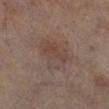Assessment:
The lesion was photographed on a routine skin check and not biopsied; there is no pathology result.
Image and clinical context:
From the left lower leg. A 15 mm close-up extracted from a 3D total-body photography capture. The recorded lesion diameter is about 4.5 mm. This is a cross-polarized tile. Automated image analysis of the tile measured a lesion area of about 14 mm², an eccentricity of roughly 0.6, and two-axis asymmetry of about 0.25. The software also gave an average lesion color of about L≈36 a*≈13 b*≈19 (CIELAB) and roughly 5 lightness units darker than nearby skin. And it measured internal color variation of about 4 on a 0–10 scale. A female patient, roughly 80 years of age.